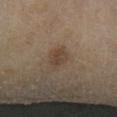| feature | finding |
|---|---|
| biopsy status | no biopsy performed (imaged during a skin exam) |
| illumination | cross-polarized |
| lesion size | ~3 mm (longest diameter) |
| imaging modality | 15 mm crop, total-body photography |
| anatomic site | the leg |
| TBP lesion metrics | a mean CIELAB color near L≈37 a*≈13 b*≈23, roughly 7 lightness units darker than nearby skin, and a normalized lesion–skin contrast near 6.5; lesion-presence confidence of about 100/100 |
| subject | female, approximately 60 years of age |